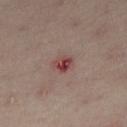No biopsy was performed on this lesion — it was imaged during a full skin examination and was not determined to be concerning.
Approximately 2.5 mm at its widest.
An algorithmic analysis of the crop reported a mean CIELAB color near L≈43 a*≈26 b*≈21 and a normalized border contrast of about 9. And it measured a border-irregularity index near 2.5/10, internal color variation of about 5.5 on a 0–10 scale, and radial color variation of about 2.
The tile uses cross-polarized illumination.
The patient is a female approximately 55 years of age.
The lesion is located on the right thigh.
A close-up tile cropped from a whole-body skin photograph, about 15 mm across.